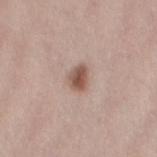Impression:
The lesion was photographed on a routine skin check and not biopsied; there is no pathology result.
Image and clinical context:
The recorded lesion diameter is about 3 mm. Cropped from a total-body skin-imaging series; the visible field is about 15 mm. The lesion is located on the left thigh. The lesion-visualizer software estimated a footprint of about 5 mm² and two-axis asymmetry of about 0.25. The analysis additionally found a nevus-likeness score of about 95/100 and a detector confidence of about 100 out of 100 that the crop contains a lesion. Captured under white-light illumination. The subject is a female in their 40s.